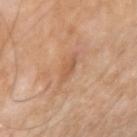Recorded during total-body skin imaging; not selected for excision or biopsy.
A roughly 15 mm field-of-view crop from a total-body skin photograph.
A male subject approximately 65 years of age.
The lesion is on the right upper arm.